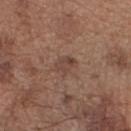Case summary:
• acquisition · ~15 mm tile from a whole-body skin photo
• lighting · white-light illumination
• subject · male, aged 68–72
• automated metrics · a footprint of about 5 mm², an eccentricity of roughly 0.65, and a shape-asymmetry score of about 0.2 (0 = symmetric); a mean CIELAB color near L≈43 a*≈18 b*≈25, about 7 CIELAB-L* units darker than the surrounding skin, and a normalized border contrast of about 6; a nevus-likeness score of about 0/100 and lesion-presence confidence of about 100/100
• size · ~3 mm (longest diameter)
• body site · the right forearm